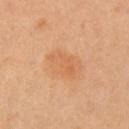{"biopsy_status": "not biopsied; imaged during a skin examination", "lesion_size": {"long_diameter_mm_approx": 4.0}, "site": "left arm", "patient": {"sex": "female", "age_approx": 40}, "automated_metrics": {"area_mm2_approx": 9.0, "eccentricity": 0.75, "shape_asymmetry": 0.2, "cielab_L": 63, "cielab_a": 24, "cielab_b": 40, "vs_skin_contrast_norm": 5.0, "nevus_likeness_0_100": 10, "lesion_detection_confidence_0_100": 100}, "lighting": "cross-polarized", "image": {"source": "total-body photography crop", "field_of_view_mm": 15}}